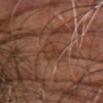The lesion was tiled from a total-body skin photograph and was not biopsied.
The patient is a male aged 58–62.
Located on the arm.
Cropped from a total-body skin-imaging series; the visible field is about 15 mm.